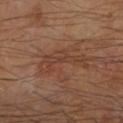Case summary:
– notes — catalogued during a skin exam; not biopsied
– image — ~15 mm tile from a whole-body skin photo
– anatomic site — the right forearm
– patient — male, roughly 65 years of age
– automated lesion analysis — a shape eccentricity near 0.9 and two-axis asymmetry of about 0.7; a lesion color around L≈38 a*≈19 b*≈27 in CIELAB, a lesion–skin lightness drop of about 6, and a normalized lesion–skin contrast near 5.5
– lighting — cross-polarized illumination
– diameter — about 6 mm